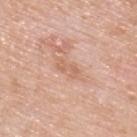Q: Was this lesion biopsied?
A: no biopsy performed (imaged during a skin exam)
Q: How was this image acquired?
A: 15 mm crop, total-body photography
Q: Patient demographics?
A: male, aged approximately 80
Q: How was the tile lit?
A: white-light illumination
Q: Automated lesion metrics?
A: a lesion area of about 3 mm², an outline eccentricity of about 0.9 (0 = round, 1 = elongated), and a shape-asymmetry score of about 0.35 (0 = symmetric); a lesion color around L≈63 a*≈22 b*≈32 in CIELAB and a normalized lesion–skin contrast near 5; a border-irregularity index near 4.5/10 and a color-variation rating of about 0/10
Q: Lesion location?
A: the upper back